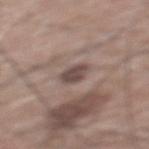A roughly 15 mm field-of-view crop from a total-body skin photograph.
On the back.
The patient is a male aged 58 to 62.
The tile uses white-light illumination.